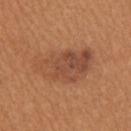{
  "biopsy_status": "not biopsied; imaged during a skin examination"
}